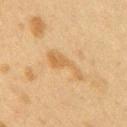patient: female, in their 40s | body site: the right upper arm | tile lighting: cross-polarized | image source: total-body-photography crop, ~15 mm field of view.